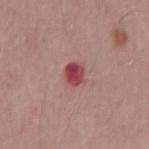Captured during whole-body skin photography for melanoma surveillance; the lesion was not biopsied.